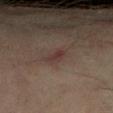follow-up: total-body-photography surveillance lesion; no biopsy | lesion diameter: ≈2.5 mm | subject: male, in their mid-60s | illumination: cross-polarized illumination | body site: the right lower leg | acquisition: ~15 mm crop, total-body skin-cancer survey.